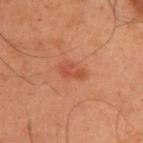Imaged during a routine full-body skin examination; the lesion was not biopsied and no histopathology is available. Cropped from a total-body skin-imaging series; the visible field is about 15 mm. A male subject, aged 53 to 57. On the back.